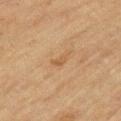Impression: Captured during whole-body skin photography for melanoma surveillance; the lesion was not biopsied. Context: A 15 mm close-up extracted from a 3D total-body photography capture. From the left upper arm. A male patient aged approximately 70.